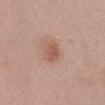notes=total-body-photography surveillance lesion; no biopsy | subject=female, aged 38–42 | image-analysis metrics=a border-irregularity rating of about 2/10 and radial color variation of about 0.5; a classifier nevus-likeness of about 75/100 and lesion-presence confidence of about 100/100 | tile lighting=white-light | lesion size=about 3 mm | site=the abdomen | acquisition=~15 mm tile from a whole-body skin photo.